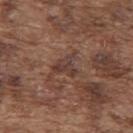Findings:
- follow-up: total-body-photography surveillance lesion; no biopsy
- subject: male, in their mid-70s
- acquisition: 15 mm crop, total-body photography
- location: the upper back